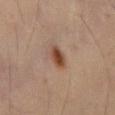{"biopsy_status": "not biopsied; imaged during a skin examination", "patient": {"sex": "male", "age_approx": 55}, "automated_metrics": {"border_irregularity_0_10": 2.5, "color_variation_0_10": 3.0, "peripheral_color_asymmetry": 1.0, "lesion_detection_confidence_0_100": 100}, "site": "abdomen", "image": {"source": "total-body photography crop", "field_of_view_mm": 15}, "lesion_size": {"long_diameter_mm_approx": 3.5}}